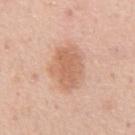subject: male, roughly 55 years of age
TBP lesion metrics: a within-lesion color-variation index near 3/10 and peripheral color asymmetry of about 1
image source: 15 mm crop, total-body photography
lesion size: ≈5 mm
body site: the abdomen